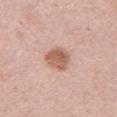<lesion>
<biopsy_status>not biopsied; imaged during a skin examination</biopsy_status>
<lesion_size>
  <long_diameter_mm_approx>3.5</long_diameter_mm_approx>
</lesion_size>
<patient>
  <sex>female</sex>
  <age_approx>50</age_approx>
</patient>
<automated_metrics>
  <area_mm2_approx>7.5</area_mm2_approx>
  <eccentricity>0.55</eccentricity>
  <shape_asymmetry>0.25</shape_asymmetry>
  <cielab_L>60</cielab_L>
  <cielab_a>22</cielab_a>
  <cielab_b>29</cielab_b>
  <vs_skin_darker_L>12.0</vs_skin_darker_L>
  <vs_skin_contrast_norm>8.5</vs_skin_contrast_norm>
  <nevus_likeness_0_100>70</nevus_likeness_0_100>
  <lesion_detection_confidence_0_100>100</lesion_detection_confidence_0_100>
</automated_metrics>
<lighting>white-light</lighting>
<site>left upper arm</site>
<image>
  <source>total-body photography crop</source>
  <field_of_view_mm>15</field_of_view_mm>
</image>
</lesion>